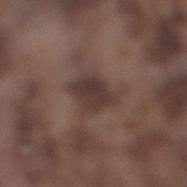Assessment:
The lesion was photographed on a routine skin check and not biopsied; there is no pathology result.
Context:
A 15 mm crop from a total-body photograph taken for skin-cancer surveillance. A male subject about 75 years old. The tile uses white-light illumination. Located on the right lower leg.